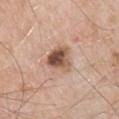Impression: No biopsy was performed on this lesion — it was imaged during a full skin examination and was not determined to be concerning. Image and clinical context: A male subject aged around 55. The recorded lesion diameter is about 3.5 mm. The lesion is on the left upper arm. A region of skin cropped from a whole-body photographic capture, roughly 15 mm wide. The tile uses white-light illumination.